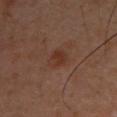This lesion was catalogued during total-body skin photography and was not selected for biopsy. A close-up tile cropped from a whole-body skin photograph, about 15 mm across. A male subject, in their 40s. On the front of the torso.